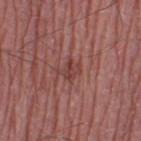Assessment:
Recorded during total-body skin imaging; not selected for excision or biopsy.
Clinical summary:
The lesion is located on the left thigh. The recorded lesion diameter is about 2.5 mm. A male patient, aged approximately 75. Cropped from a total-body skin-imaging series; the visible field is about 15 mm. Captured under white-light illumination.